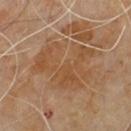{"biopsy_status": "not biopsied; imaged during a skin examination", "image": {"source": "total-body photography crop", "field_of_view_mm": 15}, "patient": {"sex": "male", "age_approx": 60}, "automated_metrics": {"area_mm2_approx": 30.0, "eccentricity": 0.6, "shape_asymmetry": 0.4, "border_irregularity_0_10": 8.0, "color_variation_0_10": 3.0, "peripheral_color_asymmetry": 1.0}, "site": "upper back"}